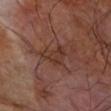Impression:
The lesion was photographed on a routine skin check and not biopsied; there is no pathology result.
Acquisition and patient details:
A male patient, aged around 70. From the left forearm. Cropped from a total-body skin-imaging series; the visible field is about 15 mm.